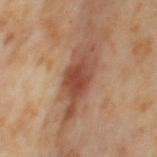Findings:
- patient: female, aged 53 to 57
- acquisition: ~15 mm tile from a whole-body skin photo
- location: the left thigh
- lighting: cross-polarized